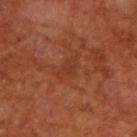Imaged during a routine full-body skin examination; the lesion was not biopsied and no histopathology is available.
An algorithmic analysis of the crop reported a mean CIELAB color near L≈35 a*≈27 b*≈32, a lesion–skin lightness drop of about 5, and a lesion-to-skin contrast of about 4.5 (normalized; higher = more distinct).
On the upper back.
A lesion tile, about 15 mm wide, cut from a 3D total-body photograph.
A male subject about 65 years old.
Measured at roughly 3 mm in maximum diameter.
This is a cross-polarized tile.